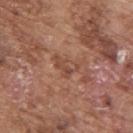illumination: white-light illumination | subject: male, aged approximately 75 | image: 15 mm crop, total-body photography | body site: the upper back | automated lesion analysis: a footprint of about 4.5 mm², an outline eccentricity of about 0.8 (0 = round, 1 = elongated), and two-axis asymmetry of about 0.45; border irregularity of about 4.5 on a 0–10 scale, a within-lesion color-variation index near 5/10, and peripheral color asymmetry of about 2; a classifier nevus-likeness of about 0/100 and lesion-presence confidence of about 85/100 | lesion diameter: ≈3.5 mm.